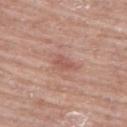notes: no biopsy performed (imaged during a skin exam)
image: 15 mm crop, total-body photography
diameter: about 2.5 mm
lighting: white-light illumination
anatomic site: the upper back
patient: female, approximately 65 years of age